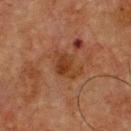imaging modality=15 mm crop, total-body photography | location=the chest | subject=male, about 75 years old.